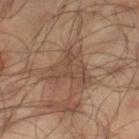Assessment: Part of a total-body skin-imaging series; this lesion was reviewed on a skin check and was not flagged for biopsy. Background: A 15 mm crop from a total-body photograph taken for skin-cancer surveillance. Longest diameter approximately 5 mm. From the right lower leg. A male subject approximately 65 years of age. Automated image analysis of the tile measured a mean CIELAB color near L≈43 a*≈16 b*≈26, a lesion–skin lightness drop of about 6, and a lesion-to-skin contrast of about 5.5 (normalized; higher = more distinct). And it measured a nevus-likeness score of about 0/100. Imaged with cross-polarized lighting.